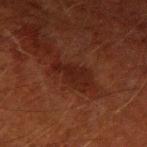Captured during whole-body skin photography for melanoma surveillance; the lesion was not biopsied. The tile uses cross-polarized illumination. A male patient, roughly 50 years of age. Located on the right upper arm. This image is a 15 mm lesion crop taken from a total-body photograph.